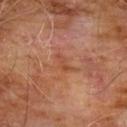Clinical impression: Part of a total-body skin-imaging series; this lesion was reviewed on a skin check and was not flagged for biopsy. Background: About 3 mm across. The lesion-visualizer software estimated a lesion area of about 3 mm², an eccentricity of roughly 0.9, and a symmetry-axis asymmetry near 0.4. It also reported a border-irregularity index near 4/10, a color-variation rating of about 0/10, and radial color variation of about 0. The tile uses cross-polarized illumination. Cropped from a total-body skin-imaging series; the visible field is about 15 mm. The lesion is on the chest. The subject is a male aged around 60.